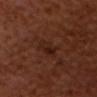Acquisition and patient details: A 15 mm close-up extracted from a 3D total-body photography capture. Automated image analysis of the tile measured border irregularity of about 3.5 on a 0–10 scale, internal color variation of about 4.5 on a 0–10 scale, and radial color variation of about 2. The analysis additionally found a classifier nevus-likeness of about 5/100 and a lesion-detection confidence of about 100/100. The lesion is on the head or neck. The subject is a male roughly 65 years of age.